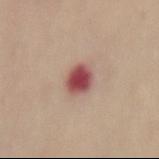Captured during whole-body skin photography for melanoma surveillance; the lesion was not biopsied. Imaged with white-light lighting. The lesion is located on the mid back. The recorded lesion diameter is about 3 mm. A female patient, aged 53–57. A 15 mm close-up tile from a total-body photography series done for melanoma screening.